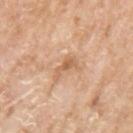follow-up=no biopsy performed (imaged during a skin exam) | illumination=white-light | diameter=≈3 mm | acquisition=15 mm crop, total-body photography | body site=the left upper arm | subject=female, aged 73 to 77.